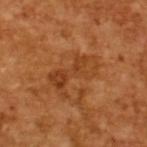notes — imaged on a skin check; not biopsied
acquisition — total-body-photography crop, ~15 mm field of view
lesion size — ~5.5 mm (longest diameter)
patient — male, in their mid- to late 60s